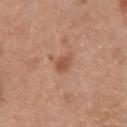biopsy status: catalogued during a skin exam; not biopsied | image-analysis metrics: a border-irregularity rating of about 2.5/10 and a color-variation rating of about 1.5/10; lesion-presence confidence of about 100/100 | location: the right forearm | acquisition: ~15 mm crop, total-body skin-cancer survey | patient: female, roughly 60 years of age | size: ≈2.5 mm | illumination: white-light.